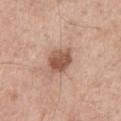lighting: white-light illumination; body site: the chest; subject: male, approximately 55 years of age; lesion size: ≈4 mm; imaging modality: total-body-photography crop, ~15 mm field of view.